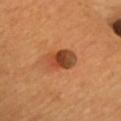The lesion was photographed on a routine skin check and not biopsied; there is no pathology result.
Longest diameter approximately 4.5 mm.
A 15 mm close-up extracted from a 3D total-body photography capture.
The lesion is on the chest.
A male subject aged approximately 65.
The tile uses cross-polarized illumination.